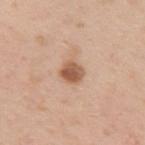Assessment:
The lesion was photographed on a routine skin check and not biopsied; there is no pathology result.
Image and clinical context:
On the left upper arm. A male subject in their mid- to late 30s. Cropped from a whole-body photographic skin survey; the tile spans about 15 mm.